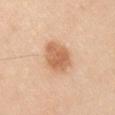This lesion was catalogued during total-body skin photography and was not selected for biopsy.
On the left upper arm.
The recorded lesion diameter is about 4.5 mm.
A male patient about 35 years old.
Imaged with white-light lighting.
A 15 mm close-up extracted from a 3D total-body photography capture.